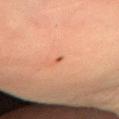Q: Was a biopsy performed?
A: no biopsy performed (imaged during a skin exam)
Q: Who is the patient?
A: female, aged approximately 30
Q: How was this image acquired?
A: ~15 mm tile from a whole-body skin photo
Q: Where on the body is the lesion?
A: the left forearm